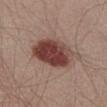The tile uses white-light illumination. A male patient, aged around 55. Cropped from a total-body skin-imaging series; the visible field is about 15 mm. Automated tile analysis of the lesion measured a lesion color around L≈42 a*≈22 b*≈23 in CIELAB, roughly 15 lightness units darker than nearby skin, and a normalized lesion–skin contrast near 11.5. It also reported an automated nevus-likeness rating near 100 out of 100 and a detector confidence of about 100 out of 100 that the crop contains a lesion. About 6.5 mm across. The lesion is on the left thigh.